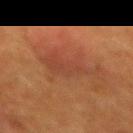Q: Was a biopsy performed?
A: imaged on a skin check; not biopsied
Q: What is the imaging modality?
A: ~15 mm crop, total-body skin-cancer survey
Q: Lesion location?
A: the mid back
Q: How large is the lesion?
A: ≈5.5 mm
Q: Illumination type?
A: cross-polarized
Q: Patient demographics?
A: female, approximately 50 years of age